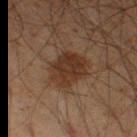workup: total-body-photography surveillance lesion; no biopsy | diameter: ~4 mm (longest diameter) | imaging modality: total-body-photography crop, ~15 mm field of view | anatomic site: the left thigh | subject: male, approximately 60 years of age.